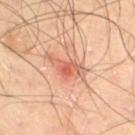- biopsy status — total-body-photography surveillance lesion; no biopsy
- lighting — cross-polarized
- diameter — about 5 mm
- subject — male, aged 68 to 72
- anatomic site — the right thigh
- acquisition — 15 mm crop, total-body photography
- automated metrics — a mean CIELAB color near L≈61 a*≈27 b*≈33, roughly 11 lightness units darker than nearby skin, and a normalized lesion–skin contrast near 7